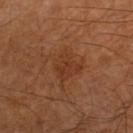Impression:
Recorded during total-body skin imaging; not selected for excision or biopsy.
Context:
Measured at roughly 3.5 mm in maximum diameter. The lesion is located on the leg. This is a cross-polarized tile. A male patient, in their mid- to late 60s. The lesion-visualizer software estimated a classifier nevus-likeness of about 5/100 and a lesion-detection confidence of about 100/100. A 15 mm close-up tile from a total-body photography series done for melanoma screening.